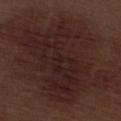Notes:
• biopsy status — imaged on a skin check; not biopsied
• site — the right thigh
• image source — total-body-photography crop, ~15 mm field of view
• patient — male, roughly 70 years of age